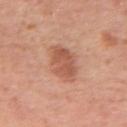Q: Was a biopsy performed?
A: imaged on a skin check; not biopsied
Q: What kind of image is this?
A: ~15 mm crop, total-body skin-cancer survey
Q: Automated lesion metrics?
A: a symmetry-axis asymmetry near 0.2; a nevus-likeness score of about 80/100 and lesion-presence confidence of about 100/100
Q: How large is the lesion?
A: ~4 mm (longest diameter)
Q: Illumination type?
A: white-light
Q: What is the anatomic site?
A: the arm
Q: Who is the patient?
A: female, approximately 55 years of age